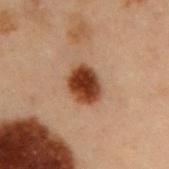Clinical impression:
Part of a total-body skin-imaging series; this lesion was reviewed on a skin check and was not flagged for biopsy.
Acquisition and patient details:
A male patient aged 53–57. A close-up tile cropped from a whole-body skin photograph, about 15 mm across. The lesion is located on the right upper arm.